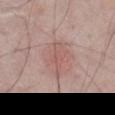Assessment: This lesion was catalogued during total-body skin photography and was not selected for biopsy. Clinical summary: About 4 mm across. Imaged with white-light lighting. A roughly 15 mm field-of-view crop from a total-body skin photograph. A male subject aged approximately 75. The lesion is located on the abdomen.